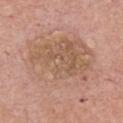Part of a total-body skin-imaging series; this lesion was reviewed on a skin check and was not flagged for biopsy. Located on the front of the torso. The patient is a male in their mid-70s. A lesion tile, about 15 mm wide, cut from a 3D total-body photograph.